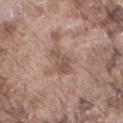The lesion was photographed on a routine skin check and not biopsied; there is no pathology result.
A roughly 15 mm field-of-view crop from a total-body skin photograph.
The tile uses white-light illumination.
The total-body-photography lesion software estimated a mean CIELAB color near L≈53 a*≈17 b*≈24, roughly 9 lightness units darker than nearby skin, and a normalized lesion–skin contrast near 6.5. It also reported a border-irregularity rating of about 4.5/10, a within-lesion color-variation index near 2.5/10, and a peripheral color-asymmetry measure near 0.5. The analysis additionally found an automated nevus-likeness rating near 0 out of 100 and a lesion-detection confidence of about 100/100.
The lesion's longest dimension is about 4.5 mm.
From the leg.
A male subject roughly 75 years of age.